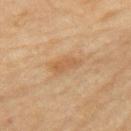  biopsy_status: not biopsied; imaged during a skin examination
  lighting: cross-polarized
  patient:
    sex: female
    age_approx: 60
  site: left upper arm
  lesion_size:
    long_diameter_mm_approx: 3.5
  automated_metrics:
    area_mm2_approx: 5.5
    eccentricity: 0.85
    cielab_L: 53
    cielab_a: 18
    cielab_b: 35
    vs_skin_darker_L: 7.0
    vs_skin_contrast_norm: 5.5
  image:
    source: total-body photography crop
    field_of_view_mm: 15